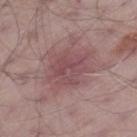Assessment: Captured during whole-body skin photography for melanoma surveillance; the lesion was not biopsied. Clinical summary: The lesion's longest dimension is about 6 mm. Automated tile analysis of the lesion measured a footprint of about 20 mm². The software also gave a color-variation rating of about 3.5/10. This is a white-light tile. A male patient aged approximately 65. On the left thigh. Cropped from a total-body skin-imaging series; the visible field is about 15 mm.